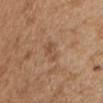Imaged during a routine full-body skin examination; the lesion was not biopsied and no histopathology is available.
Imaged with white-light lighting.
A male subject aged approximately 70.
A close-up tile cropped from a whole-body skin photograph, about 15 mm across.
About 3 mm across.
From the chest.